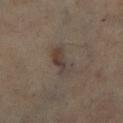This lesion was catalogued during total-body skin photography and was not selected for biopsy. A 15 mm close-up tile from a total-body photography series done for melanoma screening. The recorded lesion diameter is about 4 mm. A female patient aged approximately 60. The lesion is on the right lower leg.